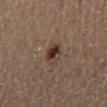Part of a total-body skin-imaging series; this lesion was reviewed on a skin check and was not flagged for biopsy. Located on the mid back. A male patient, roughly 55 years of age. The recorded lesion diameter is about 3.5 mm. This is a cross-polarized tile. The total-body-photography lesion software estimated an area of roughly 5.5 mm², an outline eccentricity of about 0.8 (0 = round, 1 = elongated), and two-axis asymmetry of about 0.2. It also reported about 10 CIELAB-L* units darker than the surrounding skin. A region of skin cropped from a whole-body photographic capture, roughly 15 mm wide.